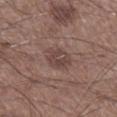follow-up: imaged on a skin check; not biopsied | location: the right lower leg | imaging modality: ~15 mm crop, total-body skin-cancer survey | automated lesion analysis: an area of roughly 6.5 mm², an outline eccentricity of about 0.65 (0 = round, 1 = elongated), and two-axis asymmetry of about 0.25; an average lesion color of about L≈43 a*≈17 b*≈21 (CIELAB), roughly 8 lightness units darker than nearby skin, and a lesion-to-skin contrast of about 6.5 (normalized; higher = more distinct); border irregularity of about 2.5 on a 0–10 scale, a within-lesion color-variation index near 4/10, and a peripheral color-asymmetry measure near 1.5; a nevus-likeness score of about 5/100 and lesion-presence confidence of about 100/100 | patient: male, aged around 60.